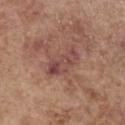biopsy status=imaged on a skin check; not biopsied